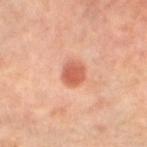The lesion was photographed on a routine skin check and not biopsied; there is no pathology result. A 15 mm close-up extracted from a 3D total-body photography capture. A female subject roughly 65 years of age. Measured at roughly 3 mm in maximum diameter. This is a cross-polarized tile. The lesion is on the right leg. Automated tile analysis of the lesion measured an area of roughly 5.5 mm², a shape eccentricity near 0.55, and a shape-asymmetry score of about 0.2 (0 = symmetric). The analysis additionally found border irregularity of about 1.5 on a 0–10 scale and radial color variation of about 1. The software also gave a nevus-likeness score of about 100/100.